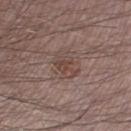workup: total-body-photography surveillance lesion; no biopsy | site: the right thigh | image: ~15 mm tile from a whole-body skin photo | size: about 3 mm | patient: male, about 70 years old | lighting: white-light illumination.